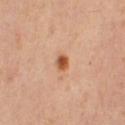Impression: No biopsy was performed on this lesion — it was imaged during a full skin examination and was not determined to be concerning. Acquisition and patient details: The lesion is located on the left thigh. About 2.5 mm across. Imaged with cross-polarized lighting. A 15 mm crop from a total-body photograph taken for skin-cancer surveillance. The lesion-visualizer software estimated an automated nevus-likeness rating near 100 out of 100 and a detector confidence of about 100 out of 100 that the crop contains a lesion. A female subject, about 40 years old.